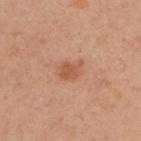follow-up: imaged on a skin check; not biopsied | diameter: ~3 mm (longest diameter) | TBP lesion metrics: an outline eccentricity of about 0.75 (0 = round, 1 = elongated) and a symmetry-axis asymmetry near 0.3; a nevus-likeness score of about 50/100 and a lesion-detection confidence of about 100/100 | image source: 15 mm crop, total-body photography | subject: male, aged around 45 | site: the upper back | lighting: cross-polarized.